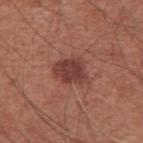Clinical impression:
No biopsy was performed on this lesion — it was imaged during a full skin examination and was not determined to be concerning.
Background:
Located on the right upper arm. This image is a 15 mm lesion crop taken from a total-body photograph. A male patient, aged around 60.